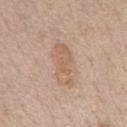A male patient approximately 60 years of age. The lesion's longest dimension is about 7 mm. The lesion is on the mid back. Imaged with white-light lighting. A region of skin cropped from a whole-body photographic capture, roughly 15 mm wide.